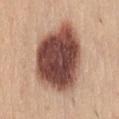The lesion was tiled from a total-body skin photograph and was not biopsied. Longest diameter approximately 9 mm. A 15 mm close-up extracted from a 3D total-body photography capture. Imaged with white-light lighting. A female subject, in their mid-20s. Automated image analysis of the tile measured a lesion area of about 44 mm² and a shape-asymmetry score of about 0.2 (0 = symmetric). The lesion is located on the abdomen.